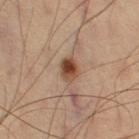Impression:
No biopsy was performed on this lesion — it was imaged during a full skin examination and was not determined to be concerning.